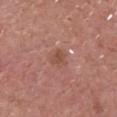  biopsy_status: not biopsied; imaged during a skin examination
  automated_metrics:
    area_mm2_approx: 3.5
    shape_asymmetry: 0.35
    border_irregularity_0_10: 3.5
    peripheral_color_asymmetry: 0.5
  image:
    source: total-body photography crop
    field_of_view_mm: 15
  patient:
    sex: male
    age_approx: 70
  site: head or neck
  lesion_size:
    long_diameter_mm_approx: 2.5
  lighting: white-light